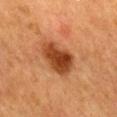Part of a total-body skin-imaging series; this lesion was reviewed on a skin check and was not flagged for biopsy.
Located on the mid back.
Approximately 5 mm at its widest.
Cropped from a whole-body photographic skin survey; the tile spans about 15 mm.
A female patient, in their mid-50s.
Imaged with cross-polarized lighting.